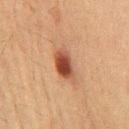Q: Automated lesion metrics?
A: a footprint of about 7.5 mm² and a symmetry-axis asymmetry near 0.25; a lesion color around L≈37 a*≈22 b*≈27 in CIELAB and a normalized border contrast of about 11; a classifier nevus-likeness of about 100/100 and a lesion-detection confidence of about 100/100
Q: How was the tile lit?
A: cross-polarized illumination
Q: What is the anatomic site?
A: the chest
Q: What is the imaging modality?
A: ~15 mm crop, total-body skin-cancer survey
Q: Patient demographics?
A: male, in their 60s